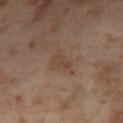Part of a total-body skin-imaging series; this lesion was reviewed on a skin check and was not flagged for biopsy. A region of skin cropped from a whole-body photographic capture, roughly 15 mm wide. From the right thigh. A female subject, about 55 years old.